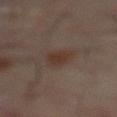Imaged during a routine full-body skin examination; the lesion was not biopsied and no histopathology is available.
Automated image analysis of the tile measured an area of roughly 5.5 mm², a shape eccentricity near 0.7, and a shape-asymmetry score of about 0.2 (0 = symmetric). The analysis additionally found a mean CIELAB color near L≈31 a*≈14 b*≈21 and a normalized lesion–skin contrast near 7.
Located on the abdomen.
A region of skin cropped from a whole-body photographic capture, roughly 15 mm wide.
A male patient aged approximately 60.
The recorded lesion diameter is about 3 mm.
The tile uses cross-polarized illumination.